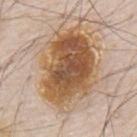Assessment:
Part of a total-body skin-imaging series; this lesion was reviewed on a skin check and was not flagged for biopsy.
Background:
A 15 mm close-up tile from a total-body photography series done for melanoma screening. From the mid back. A male patient, aged 78–82.